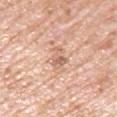biopsy status: total-body-photography surveillance lesion; no biopsy
image: total-body-photography crop, ~15 mm field of view
illumination: white-light illumination
subject: male, aged around 80
automated metrics: an area of roughly 4.5 mm² and a shape-asymmetry score of about 0.35 (0 = symmetric); an average lesion color of about L≈64 a*≈21 b*≈30 (CIELAB), a lesion–skin lightness drop of about 10, and a normalized border contrast of about 6
location: the left upper arm